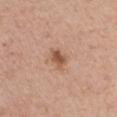Clinical impression:
Recorded during total-body skin imaging; not selected for excision or biopsy.
Context:
The lesion's longest dimension is about 3 mm. The lesion is located on the left upper arm. This image is a 15 mm lesion crop taken from a total-body photograph. A male patient, in their mid- to late 70s. An algorithmic analysis of the crop reported a footprint of about 4 mm², a shape eccentricity near 0.75, and a symmetry-axis asymmetry near 0.3. It also reported a mean CIELAB color near L≈54 a*≈22 b*≈31, roughly 12 lightness units darker than nearby skin, and a normalized border contrast of about 8. And it measured a nevus-likeness score of about 80/100 and lesion-presence confidence of about 100/100.